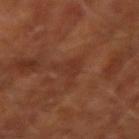| feature | finding |
|---|---|
| biopsy status | imaged on a skin check; not biopsied |
| patient | male, aged around 65 |
| image source | ~15 mm tile from a whole-body skin photo |
| illumination | cross-polarized |
| location | the right forearm |